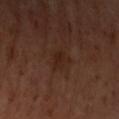biopsy_status: not biopsied; imaged during a skin examination
patient:
  sex: female
  age_approx: 50
image:
  source: total-body photography crop
  field_of_view_mm: 15
site: left upper arm